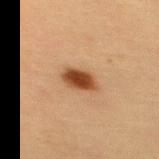The lesion was photographed on a routine skin check and not biopsied; there is no pathology result.
The lesion is located on the upper back.
A female patient, about 65 years old.
Captured under cross-polarized illumination.
The lesion's longest dimension is about 3.5 mm.
The total-body-photography lesion software estimated a lesion area of about 6.5 mm², an eccentricity of roughly 0.75, and a symmetry-axis asymmetry near 0.15. And it measured a border-irregularity rating of about 1.5/10, internal color variation of about 3.5 on a 0–10 scale, and a peripheral color-asymmetry measure near 1.
This image is a 15 mm lesion crop taken from a total-body photograph.